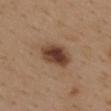biopsy status: imaged on a skin check; not biopsied
location: the back
image: ~15 mm tile from a whole-body skin photo
patient: female, in their 40s